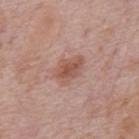No biopsy was performed on this lesion — it was imaged during a full skin examination and was not determined to be concerning. Automated image analysis of the tile measured an average lesion color of about L≈53 a*≈22 b*≈27 (CIELAB), about 10 CIELAB-L* units darker than the surrounding skin, and a normalized border contrast of about 7. And it measured border irregularity of about 2.5 on a 0–10 scale, a within-lesion color-variation index near 3/10, and a peripheral color-asymmetry measure near 1. A 15 mm close-up extracted from a 3D total-body photography capture. The patient is a male aged 73–77. Imaged with white-light lighting. Measured at roughly 3.5 mm in maximum diameter. On the mid back.